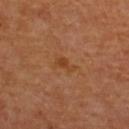| feature | finding |
|---|---|
| biopsy status | imaged on a skin check; not biopsied |
| imaging modality | ~15 mm tile from a whole-body skin photo |
| subject | female, aged 48–52 |
| site | the upper back |